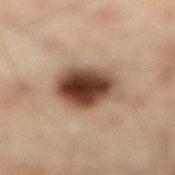A male subject, in their mid- to late 40s. A 15 mm close-up tile from a total-body photography series done for melanoma screening. From the right lower leg. Captured under cross-polarized illumination. The lesion was biopsied, and histopathology showed a dysplastic (Clark) nevus, classified as a benign skin lesion.